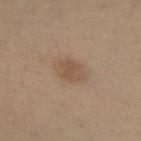The lesion was photographed on a routine skin check and not biopsied; there is no pathology result.